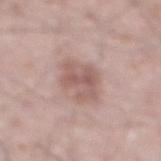This lesion was catalogued during total-body skin photography and was not selected for biopsy.
From the abdomen.
A male subject in their mid- to late 60s.
Captured under white-light illumination.
An algorithmic analysis of the crop reported a shape eccentricity near 0.7 and a symmetry-axis asymmetry near 0.25. The analysis additionally found a mean CIELAB color near L≈57 a*≈19 b*≈22, about 10 CIELAB-L* units darker than the surrounding skin, and a normalized lesion–skin contrast near 6.5. It also reported a border-irregularity rating of about 3.5/10. The software also gave lesion-presence confidence of about 100/100.
A 15 mm close-up tile from a total-body photography series done for melanoma screening.